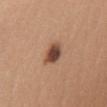Q: Was this lesion biopsied?
A: total-body-photography surveillance lesion; no biopsy
Q: Where on the body is the lesion?
A: the front of the torso
Q: Automated lesion metrics?
A: a lesion color around L≈47 a*≈21 b*≈29 in CIELAB, about 15 CIELAB-L* units darker than the surrounding skin, and a normalized lesion–skin contrast near 11; a peripheral color-asymmetry measure near 1.5
Q: How was the tile lit?
A: white-light illumination
Q: Who is the patient?
A: female, roughly 55 years of age
Q: Lesion size?
A: ≈3 mm
Q: What kind of image is this?
A: 15 mm crop, total-body photography